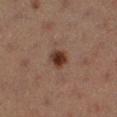A female subject aged around 40. A 15 mm close-up extracted from a 3D total-body photography capture. Approximately 2.5 mm at its widest. The tile uses cross-polarized illumination. Located on the left lower leg.